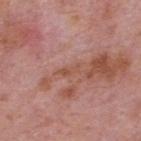Recorded during total-body skin imaging; not selected for excision or biopsy. An algorithmic analysis of the crop reported a lesion color around L≈52 a*≈23 b*≈29 in CIELAB and a normalized border contrast of about 6. The analysis additionally found a border-irregularity index near 5/10, a within-lesion color-variation index near 0/10, and radial color variation of about 0. The analysis additionally found a nevus-likeness score of about 0/100 and a detector confidence of about 65 out of 100 that the crop contains a lesion. A region of skin cropped from a whole-body photographic capture, roughly 15 mm wide. Captured under white-light illumination. The lesion is located on the upper back. The subject is a male aged around 75. Measured at roughly 3 mm in maximum diameter.